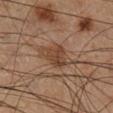The lesion was tiled from a total-body skin photograph and was not biopsied. A male subject, approximately 60 years of age. Located on the leg. Captured under cross-polarized illumination. Cropped from a whole-body photographic skin survey; the tile spans about 15 mm. Automated image analysis of the tile measured a mean CIELAB color near L≈43 a*≈18 b*≈29, roughly 8 lightness units darker than nearby skin, and a normalized border contrast of about 7. And it measured border irregularity of about 4 on a 0–10 scale, a color-variation rating of about 3.5/10, and a peripheral color-asymmetry measure near 1.5. The software also gave a classifier nevus-likeness of about 20/100 and lesion-presence confidence of about 100/100.